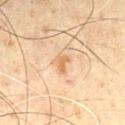biopsy status = catalogued during a skin exam; not biopsied | lesion diameter = ≈2.5 mm | subject = male, about 60 years old | lighting = cross-polarized illumination | image = total-body-photography crop, ~15 mm field of view.